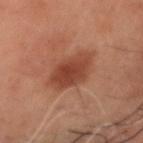Clinical impression:
The lesion was photographed on a routine skin check and not biopsied; there is no pathology result.
Clinical summary:
The lesion is on the head or neck. A 15 mm close-up tile from a total-body photography series done for melanoma screening. A male subject, aged 48–52.